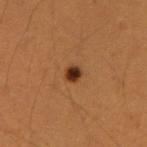Assessment: Recorded during total-body skin imaging; not selected for excision or biopsy. Image and clinical context: This is a cross-polarized tile. Approximately 2 mm at its widest. A female subject, aged 38–42. Located on the right upper arm. A 15 mm close-up tile from a total-body photography series done for melanoma screening. An algorithmic analysis of the crop reported a mean CIELAB color near L≈34 a*≈23 b*≈33, roughly 15 lightness units darker than nearby skin, and a normalized lesion–skin contrast near 13. It also reported a classifier nevus-likeness of about 100/100.